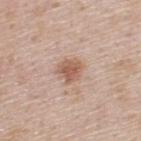This lesion was catalogued during total-body skin photography and was not selected for biopsy. The subject is a male in their mid-40s. Located on the upper back. Imaged with white-light lighting. Cropped from a whole-body photographic skin survey; the tile spans about 15 mm.